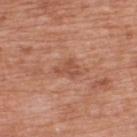Recorded during total-body skin imaging; not selected for excision or biopsy.
A male subject aged 68 to 72.
The lesion is located on the upper back.
Automated tile analysis of the lesion measured a footprint of about 4.5 mm², an outline eccentricity of about 0.7 (0 = round, 1 = elongated), and a shape-asymmetry score of about 0.55 (0 = symmetric). It also reported a border-irregularity index near 5/10, a color-variation rating of about 2/10, and radial color variation of about 1. And it measured a classifier nevus-likeness of about 0/100 and a lesion-detection confidence of about 100/100.
About 2.5 mm across.
Cropped from a total-body skin-imaging series; the visible field is about 15 mm.
This is a white-light tile.